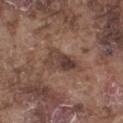{"biopsy_status": "not biopsied; imaged during a skin examination", "patient": {"sex": "male", "age_approx": 75}, "automated_metrics": {"area_mm2_approx": 9.0, "eccentricity": 0.85, "shape_asymmetry": 0.35, "cielab_L": 40, "cielab_a": 18, "cielab_b": 23, "vs_skin_darker_L": 9.0, "vs_skin_contrast_norm": 8.0, "border_irregularity_0_10": 4.0, "peripheral_color_asymmetry": 3.0}, "lesion_size": {"long_diameter_mm_approx": 4.5}, "image": {"source": "total-body photography crop", "field_of_view_mm": 15}, "site": "leg", "lighting": "white-light"}